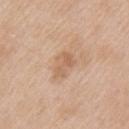workup: imaged on a skin check; not biopsied | body site: the left upper arm | image: ~15 mm crop, total-body skin-cancer survey | subject: female, aged around 40 | lighting: white-light illumination | TBP lesion metrics: a lesion area of about 5.5 mm², a shape eccentricity near 0.75, and a symmetry-axis asymmetry near 0.3; a mean CIELAB color near L≈62 a*≈19 b*≈33, about 8 CIELAB-L* units darker than the surrounding skin, and a lesion-to-skin contrast of about 5.5 (normalized; higher = more distinct); a border-irregularity index near 3/10, a color-variation rating of about 3/10, and a peripheral color-asymmetry measure near 1; a nevus-likeness score of about 0/100 and a detector confidence of about 100 out of 100 that the crop contains a lesion.